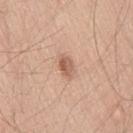Clinical impression: Part of a total-body skin-imaging series; this lesion was reviewed on a skin check and was not flagged for biopsy. Context: Cropped from a whole-body photographic skin survey; the tile spans about 15 mm. A male subject approximately 45 years of age. From the leg. An algorithmic analysis of the crop reported about 11 CIELAB-L* units darker than the surrounding skin and a normalized lesion–skin contrast near 7. Imaged with white-light lighting. About 3 mm across.